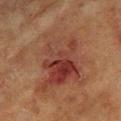Image and clinical context: From the left forearm. Measured at roughly 9.5 mm in maximum diameter. A female subject, about 80 years old. This is a cross-polarized tile. A lesion tile, about 15 mm wide, cut from a 3D total-body photograph. The total-body-photography lesion software estimated an area of roughly 43 mm² and an outline eccentricity of about 0.7 (0 = round, 1 = elongated).